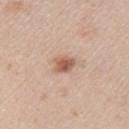The lesion was photographed on a routine skin check and not biopsied; there is no pathology result.
The subject is a male approximately 40 years of age.
About 2.5 mm across.
The tile uses white-light illumination.
Cropped from a whole-body photographic skin survey; the tile spans about 15 mm.
Automated image analysis of the tile measured a lesion color around L≈58 a*≈20 b*≈30 in CIELAB, about 13 CIELAB-L* units darker than the surrounding skin, and a lesion-to-skin contrast of about 8.5 (normalized; higher = more distinct). And it measured a border-irregularity index near 2.5/10, a color-variation rating of about 3.5/10, and peripheral color asymmetry of about 1. It also reported an automated nevus-likeness rating near 90 out of 100 and a lesion-detection confidence of about 100/100.
From the left upper arm.